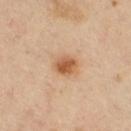workup = catalogued during a skin exam; not biopsied
automated lesion analysis = an area of roughly 6 mm², a shape eccentricity near 0.6, and a symmetry-axis asymmetry near 0.15; a lesion-detection confidence of about 100/100
subject = male, aged approximately 65
body site = the right thigh
acquisition = ~15 mm tile from a whole-body skin photo
illumination = cross-polarized illumination
lesion size = about 3 mm